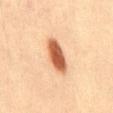Notes:
• notes · no biopsy performed (imaged during a skin exam)
• site · the abdomen
• patient · male, aged 38–42
• image source · ~15 mm tile from a whole-body skin photo